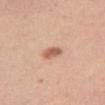workup = catalogued during a skin exam; not biopsied | lesion size = ~2.5 mm (longest diameter) | imaging modality = ~15 mm tile from a whole-body skin photo | subject = female, aged 28 to 32 | anatomic site = the right thigh.